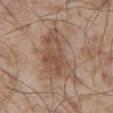Findings:
* biopsy status: total-body-photography surveillance lesion; no biopsy
* anatomic site: the abdomen
* imaging modality: total-body-photography crop, ~15 mm field of view
* illumination: white-light illumination
* diameter: about 7 mm
* patient: male, roughly 55 years of age